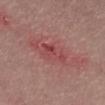This lesion was catalogued during total-body skin photography and was not selected for biopsy.
About 4 mm across.
A male patient, roughly 40 years of age.
This image is a 15 mm lesion crop taken from a total-body photograph.
On the abdomen.
This is a white-light tile.